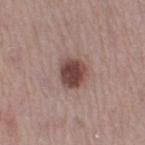Q: Was a biopsy performed?
A: imaged on a skin check; not biopsied
Q: What lighting was used for the tile?
A: white-light
Q: How was this image acquired?
A: total-body-photography crop, ~15 mm field of view
Q: What is the anatomic site?
A: the leg
Q: Who is the patient?
A: female, aged around 40
Q: Lesion size?
A: ≈3.5 mm